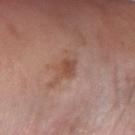Impression:
This lesion was catalogued during total-body skin photography and was not selected for biopsy.
Clinical summary:
Located on the right forearm. A 15 mm crop from a total-body photograph taken for skin-cancer surveillance. The patient is a female approximately 75 years of age. The tile uses white-light illumination. The total-body-photography lesion software estimated border irregularity of about 2 on a 0–10 scale and a peripheral color-asymmetry measure near 1.